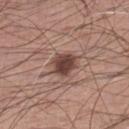  biopsy_status: not biopsied; imaged during a skin examination
  patient:
    sex: male
    age_approx: 60
  image:
    source: total-body photography crop
    field_of_view_mm: 15
  automated_metrics:
    area_mm2_approx: 6.5
    eccentricity: 0.55
    shape_asymmetry: 0.25
    cielab_L: 43
    cielab_a: 20
    cielab_b: 23
    vs_skin_darker_L: 15.0
    vs_skin_contrast_norm: 11.0
    color_variation_0_10: 3.0
    peripheral_color_asymmetry: 1.0
    nevus_likeness_0_100: 95
    lesion_detection_confidence_0_100: 100
  site: leg